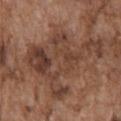The lesion was photographed on a routine skin check and not biopsied; there is no pathology result. A 15 mm close-up extracted from a 3D total-body photography capture. Automated image analysis of the tile measured an area of roughly 35 mm², an eccentricity of roughly 0.7, and a symmetry-axis asymmetry near 0.6. And it measured an average lesion color of about L≈41 a*≈19 b*≈26 (CIELAB), about 9 CIELAB-L* units darker than the surrounding skin, and a lesion-to-skin contrast of about 7.5 (normalized; higher = more distinct). The tile uses white-light illumination. The subject is a male roughly 75 years of age. On the chest.